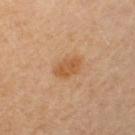Impression: Captured during whole-body skin photography for melanoma surveillance; the lesion was not biopsied. Background: A female subject aged around 50. The total-body-photography lesion software estimated an area of roughly 5.5 mm², an outline eccentricity of about 0.8 (0 = round, 1 = elongated), and two-axis asymmetry of about 0.2. It also reported an average lesion color of about L≈53 a*≈21 b*≈38 (CIELAB), roughly 8 lightness units darker than nearby skin, and a normalized lesion–skin contrast near 7. It also reported a border-irregularity index near 2/10 and internal color variation of about 2.5 on a 0–10 scale. The lesion is on the left upper arm. This image is a 15 mm lesion crop taken from a total-body photograph.